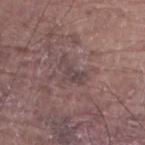This image is a 15 mm lesion crop taken from a total-body photograph.
Approximately 3.5 mm at its widest.
This is a white-light tile.
A male patient, approximately 80 years of age.
Automated tile analysis of the lesion measured an outline eccentricity of about 0.9 (0 = round, 1 = elongated). It also reported an average lesion color of about L≈43 a*≈15 b*≈15 (CIELAB), roughly 7 lightness units darker than nearby skin, and a lesion-to-skin contrast of about 6 (normalized; higher = more distinct). It also reported a within-lesion color-variation index near 1.5/10 and a peripheral color-asymmetry measure near 0.5.
From the left lower leg.